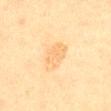No biopsy was performed on this lesion — it was imaged during a full skin examination and was not determined to be concerning. The lesion-visualizer software estimated a mean CIELAB color near L≈66 a*≈15 b*≈39 and about 6 CIELAB-L* units darker than the surrounding skin. It also reported border irregularity of about 1.5 on a 0–10 scale, internal color variation of about 2 on a 0–10 scale, and radial color variation of about 1. The analysis additionally found an automated nevus-likeness rating near 0 out of 100. Imaged with cross-polarized lighting. Located on the front of the torso. A 15 mm close-up tile from a total-body photography series done for melanoma screening. Longest diameter approximately 3.5 mm. A female subject aged around 55.